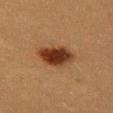Q: Is there a histopathology result?
A: no biopsy performed (imaged during a skin exam)
Q: Who is the patient?
A: female, aged 38–42
Q: What did automated image analysis measure?
A: an area of roughly 11 mm²; an average lesion color of about L≈29 a*≈20 b*≈28 (CIELAB) and roughly 14 lightness units darker than nearby skin; border irregularity of about 2 on a 0–10 scale, a within-lesion color-variation index near 4.5/10, and radial color variation of about 1.5
Q: Lesion size?
A: ~5 mm (longest diameter)
Q: What is the anatomic site?
A: the left thigh
Q: How was this image acquired?
A: total-body-photography crop, ~15 mm field of view